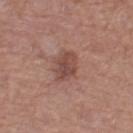Clinical impression:
Part of a total-body skin-imaging series; this lesion was reviewed on a skin check and was not flagged for biopsy.
Background:
Automated image analysis of the tile measured a within-lesion color-variation index near 3/10 and peripheral color asymmetry of about 1. The analysis additionally found a nevus-likeness score of about 5/100 and a detector confidence of about 100 out of 100 that the crop contains a lesion. The lesion is on the left thigh. A region of skin cropped from a whole-body photographic capture, roughly 15 mm wide. Approximately 3.5 mm at its widest. Captured under white-light illumination. A female subject, in their 60s.